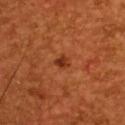The lesion was tiled from a total-body skin photograph and was not biopsied. Approximately 2 mm at its widest. Captured under cross-polarized illumination. The lesion is on the upper back. A region of skin cropped from a whole-body photographic capture, roughly 15 mm wide. A male subject, aged around 45.